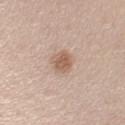| key | value |
|---|---|
| follow-up | no biopsy performed (imaged during a skin exam) |
| body site | the arm |
| patient | male, aged approximately 45 |
| automated metrics | a footprint of about 5.5 mm², an outline eccentricity of about 0.45 (0 = round, 1 = elongated), and a symmetry-axis asymmetry near 0.15; a nevus-likeness score of about 85/100 |
| imaging modality | 15 mm crop, total-body photography |
| lighting | white-light illumination |